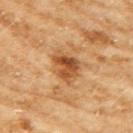Imaged during a routine full-body skin examination; the lesion was not biopsied and no histopathology is available. Located on the right upper arm. A roughly 15 mm field-of-view crop from a total-body skin photograph. A female subject, about 60 years old.